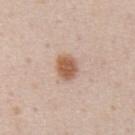follow-up = total-body-photography surveillance lesion; no biopsy
acquisition = ~15 mm crop, total-body skin-cancer survey
location = the abdomen
lighting = white-light illumination
patient = male, aged approximately 60